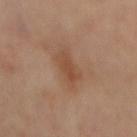Assessment: Part of a total-body skin-imaging series; this lesion was reviewed on a skin check and was not flagged for biopsy. Background: This is a cross-polarized tile. The lesion is located on the mid back. A lesion tile, about 15 mm wide, cut from a 3D total-body photograph. The recorded lesion diameter is about 4.5 mm.